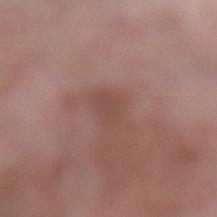<record>
  <biopsy_status>not biopsied; imaged during a skin examination</biopsy_status>
  <patient>
    <sex>female</sex>
    <age_approx>70</age_approx>
  </patient>
  <site>left lower leg</site>
  <lighting>white-light</lighting>
  <lesion_size>
    <long_diameter_mm_approx>3.0</long_diameter_mm_approx>
  </lesion_size>
  <automated_metrics>
    <area_mm2_approx>4.5</area_mm2_approx>
    <eccentricity>0.7</eccentricity>
    <shape_asymmetry>0.3</shape_asymmetry>
    <color_variation_0_10>1.5</color_variation_0_10>
    <peripheral_color_asymmetry>0.5</peripheral_color_asymmetry>
    <nevus_likeness_0_100>0</nevus_likeness_0_100>
  </automated_metrics>
  <image>
    <source>total-body photography crop</source>
    <field_of_view_mm>15</field_of_view_mm>
  </image>
</record>